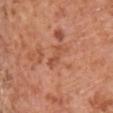Findings:
– follow-up · catalogued during a skin exam; not biopsied
– body site · the chest
– lesion diameter · ≈3 mm
– tile lighting · white-light
– patient · male, approximately 65 years of age
– image source · 15 mm crop, total-body photography